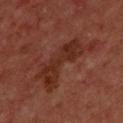Imaged during a routine full-body skin examination; the lesion was not biopsied and no histopathology is available. Approximately 8 mm at its widest. A male subject, in their mid- to late 60s. This is a cross-polarized tile. A close-up tile cropped from a whole-body skin photograph, about 15 mm across. The lesion is located on the upper back.